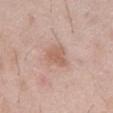Clinical impression:
Captured during whole-body skin photography for melanoma surveillance; the lesion was not biopsied.
Acquisition and patient details:
The total-body-photography lesion software estimated two-axis asymmetry of about 0.45. The software also gave a nevus-likeness score of about 10/100 and a lesion-detection confidence of about 100/100. The lesion's longest dimension is about 3 mm. A male patient, roughly 50 years of age. The tile uses white-light illumination. The lesion is located on the abdomen. A lesion tile, about 15 mm wide, cut from a 3D total-body photograph.